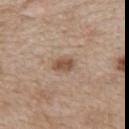Notes:
* workup: catalogued during a skin exam; not biopsied
* subject: female, aged 38 to 42
* imaging modality: ~15 mm crop, total-body skin-cancer survey
* anatomic site: the mid back